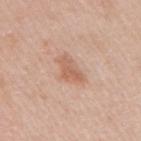The lesion was tiled from a total-body skin photograph and was not biopsied.
Longest diameter approximately 3.5 mm.
The subject is a female roughly 40 years of age.
Imaged with white-light lighting.
Automated tile analysis of the lesion measured a border-irregularity index near 3.5/10, a color-variation rating of about 2.5/10, and a peripheral color-asymmetry measure near 1. It also reported a classifier nevus-likeness of about 5/100 and a detector confidence of about 100 out of 100 that the crop contains a lesion.
A lesion tile, about 15 mm wide, cut from a 3D total-body photograph.
The lesion is on the right upper arm.